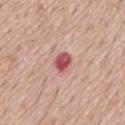No biopsy was performed on this lesion — it was imaged during a full skin examination and was not determined to be concerning. From the mid back. A male patient, in their mid- to late 50s. The tile uses white-light illumination. A roughly 15 mm field-of-view crop from a total-body skin photograph.